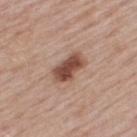No biopsy was performed on this lesion — it was imaged during a full skin examination and was not determined to be concerning. A region of skin cropped from a whole-body photographic capture, roughly 15 mm wide. This is a white-light tile. The subject is a male aged 63–67. The lesion is located on the upper back. Measured at roughly 4 mm in maximum diameter. Automated tile analysis of the lesion measured a lesion area of about 8 mm², a shape eccentricity near 0.85, and two-axis asymmetry of about 0.2. And it measured a border-irregularity index near 2.5/10, a within-lesion color-variation index near 3.5/10, and radial color variation of about 1. It also reported an automated nevus-likeness rating near 90 out of 100 and lesion-presence confidence of about 100/100.